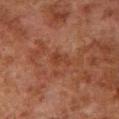Assessment: Captured during whole-body skin photography for melanoma surveillance; the lesion was not biopsied. Background: This is a cross-polarized tile. Approximately 3 mm at its widest. A male subject, approximately 80 years of age. A close-up tile cropped from a whole-body skin photograph, about 15 mm across. On the left lower leg.